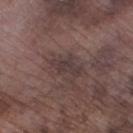biopsy_status: not biopsied; imaged during a skin examination
site: left lower leg
lighting: white-light
patient:
  sex: male
  age_approx: 75
image:
  source: total-body photography crop
  field_of_view_mm: 15